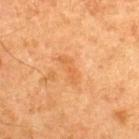follow-up = no biopsy performed (imaged during a skin exam)
diameter = ≈3.5 mm
subject = male, aged around 65
TBP lesion metrics = two-axis asymmetry of about 0.35
body site = the upper back
tile lighting = cross-polarized illumination
image source = ~15 mm tile from a whole-body skin photo